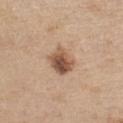Impression: No biopsy was performed on this lesion — it was imaged during a full skin examination and was not determined to be concerning. Image and clinical context: The recorded lesion diameter is about 3.5 mm. A region of skin cropped from a whole-body photographic capture, roughly 15 mm wide. The lesion is located on the chest. This is a white-light tile. A female subject aged 43 to 47.